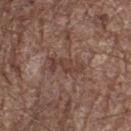Q: Was a biopsy performed?
A: total-body-photography surveillance lesion; no biopsy
Q: Where on the body is the lesion?
A: the left thigh
Q: What kind of image is this?
A: total-body-photography crop, ~15 mm field of view
Q: How was the tile lit?
A: white-light illumination
Q: How large is the lesion?
A: ~4 mm (longest diameter)
Q: Who is the patient?
A: male, in their 70s